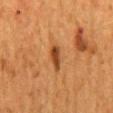No biopsy was performed on this lesion — it was imaged during a full skin examination and was not determined to be concerning. The tile uses cross-polarized illumination. The lesion is on the mid back. The patient is a female about 50 years old. About 3 mm across. A region of skin cropped from a whole-body photographic capture, roughly 15 mm wide.